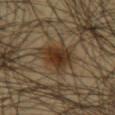Impression:
The lesion was photographed on a routine skin check and not biopsied; there is no pathology result.
Clinical summary:
Approximately 5 mm at its widest. The lesion is on the left upper arm. A roughly 15 mm field-of-view crop from a total-body skin photograph. A male patient approximately 45 years of age. This is a cross-polarized tile.